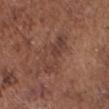{"biopsy_status": "not biopsied; imaged during a skin examination", "lesion_size": {"long_diameter_mm_approx": 5.0}, "lighting": "white-light", "site": "head or neck", "image": {"source": "total-body photography crop", "field_of_view_mm": 15}, "automated_metrics": {"cielab_L": 40, "cielab_a": 20, "cielab_b": 24, "vs_skin_darker_L": 6.0, "vs_skin_contrast_norm": 5.5}, "patient": {"sex": "male", "age_approx": 75}}